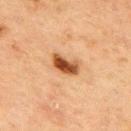Assessment:
Imaged during a routine full-body skin examination; the lesion was not biopsied and no histopathology is available.
Image and clinical context:
A male patient, aged approximately 70. An algorithmic analysis of the crop reported a border-irregularity index near 3/10, a within-lesion color-variation index near 5.5/10, and radial color variation of about 1.5. Located on the back. A 15 mm close-up tile from a total-body photography series done for melanoma screening.